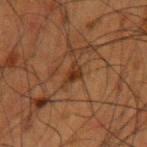Findings:
• notes: no biopsy performed (imaged during a skin exam)
• body site: the left upper arm
• lighting: cross-polarized
• image source: 15 mm crop, total-body photography
• patient: male, about 50 years old
• lesion size: ~2.5 mm (longest diameter)